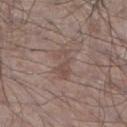No biopsy was performed on this lesion — it was imaged during a full skin examination and was not determined to be concerning. The lesion is located on the right lower leg. A 15 mm close-up tile from a total-body photography series done for melanoma screening. This is a white-light tile. A male subject, in their mid-60s. Automated tile analysis of the lesion measured an eccentricity of roughly 0.85 and two-axis asymmetry of about 0.3. It also reported a mean CIELAB color near L≈49 a*≈16 b*≈21, about 6 CIELAB-L* units darker than the surrounding skin, and a lesion-to-skin contrast of about 5 (normalized; higher = more distinct). And it measured a border-irregularity rating of about 4.5/10, a within-lesion color-variation index near 2/10, and a peripheral color-asymmetry measure near 0.5. About 4 mm across.